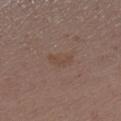{"biopsy_status": "not biopsied; imaged during a skin examination", "lighting": "white-light", "site": "right lower leg", "lesion_size": {"long_diameter_mm_approx": 3.0}, "image": {"source": "total-body photography crop", "field_of_view_mm": 15}, "patient": {"sex": "female", "age_approx": 30}}